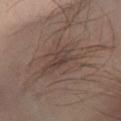Captured during whole-body skin photography for melanoma surveillance; the lesion was not biopsied.
This image is a 15 mm lesion crop taken from a total-body photograph.
Measured at roughly 11.5 mm in maximum diameter.
Automated tile analysis of the lesion measured an area of roughly 25 mm², a shape eccentricity near 0.9, and a symmetry-axis asymmetry near 0.45. It also reported a border-irregularity index near 8.5/10, a color-variation rating of about 4.5/10, and radial color variation of about 1.5. And it measured an automated nevus-likeness rating near 0 out of 100 and lesion-presence confidence of about 85/100.
This is a cross-polarized tile.
The subject is a male approximately 40 years of age.
The lesion is located on the right lower leg.